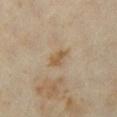Notes:
• workup — catalogued during a skin exam; not biopsied
• patient — female, in their mid- to late 30s
• image-analysis metrics — a border-irregularity index near 2.5/10, internal color variation of about 2 on a 0–10 scale, and radial color variation of about 1
• image — ~15 mm tile from a whole-body skin photo
• tile lighting — cross-polarized illumination
• lesion size — ~3 mm (longest diameter)
• location — the chest